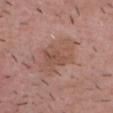The lesion is located on the head or neck.
The patient is a male aged 53–57.
This image is a 15 mm lesion crop taken from a total-body photograph.
The tile uses white-light illumination.
Automated image analysis of the tile measured a footprint of about 16 mm², a shape eccentricity near 0.75, and a shape-asymmetry score of about 0.3 (0 = symmetric). The software also gave a lesion color around L≈52 a*≈20 b*≈26 in CIELAB and roughly 7 lightness units darker than nearby skin.
About 5.5 mm across.